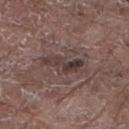Clinical impression:
Recorded during total-body skin imaging; not selected for excision or biopsy.
Clinical summary:
Automated tile analysis of the lesion measured a lesion area of about 7.5 mm², an eccentricity of roughly 0.95, and a shape-asymmetry score of about 0.4 (0 = symmetric). The software also gave a border-irregularity index near 6/10 and a color-variation rating of about 7/10. A male patient about 80 years old. A lesion tile, about 15 mm wide, cut from a 3D total-body photograph. From the left lower leg. Measured at roughly 5 mm in maximum diameter. Captured under white-light illumination.